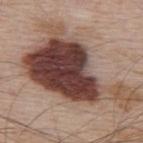{"automated_metrics": {"area_mm2_approx": 55.0, "shape_asymmetry": 0.5, "lesion_detection_confidence_0_100": 100}, "site": "upper back", "patient": {"sex": "male", "age_approx": 65}, "lighting": "white-light", "image": {"source": "total-body photography crop", "field_of_view_mm": 15}}